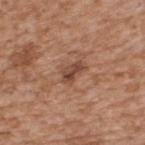tile lighting = white-light
acquisition = 15 mm crop, total-body photography
lesion diameter = ≈3 mm
image-analysis metrics = a lesion color around L≈46 a*≈22 b*≈29 in CIELAB and a normalized border contrast of about 8; a border-irregularity index near 3/10 and a color-variation rating of about 4.5/10
location = the upper back
patient = male, aged 63 to 67